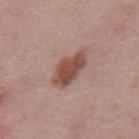The lesion was photographed on a routine skin check and not biopsied; there is no pathology result.
The lesion-visualizer software estimated a footprint of about 10 mm², an outline eccentricity of about 0.85 (0 = round, 1 = elongated), and a shape-asymmetry score of about 0.25 (0 = symmetric). The software also gave border irregularity of about 2.5 on a 0–10 scale, internal color variation of about 2.5 on a 0–10 scale, and a peripheral color-asymmetry measure near 1. And it measured an automated nevus-likeness rating near 95 out of 100 and lesion-presence confidence of about 100/100.
From the mid back.
Measured at roughly 5 mm in maximum diameter.
The subject is a male aged around 45.
Cropped from a whole-body photographic skin survey; the tile spans about 15 mm.